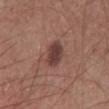Captured during whole-body skin photography for melanoma surveillance; the lesion was not biopsied. Imaged with white-light lighting. The lesion is located on the mid back. A 15 mm close-up tile from a total-body photography series done for melanoma screening. The patient is a male about 60 years old. The recorded lesion diameter is about 4 mm.